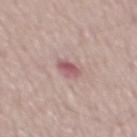Recorded during total-body skin imaging; not selected for excision or biopsy. Longest diameter approximately 2.5 mm. Cropped from a whole-body photographic skin survey; the tile spans about 15 mm. The subject is a male aged approximately 55. Imaged with white-light lighting.